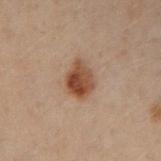Assessment: Recorded during total-body skin imaging; not selected for excision or biopsy.